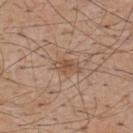Case summary:
– follow-up · catalogued during a skin exam; not biopsied
– imaging modality · ~15 mm tile from a whole-body skin photo
– size · about 2.5 mm
– tile lighting · white-light
– anatomic site · the upper back
– automated metrics · a lesion area of about 3.5 mm², a shape eccentricity near 0.8, and a shape-asymmetry score of about 0.25 (0 = symmetric); a classifier nevus-likeness of about 10/100 and a lesion-detection confidence of about 100/100
– patient · male, aged approximately 55